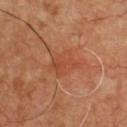Impression: Captured during whole-body skin photography for melanoma surveillance; the lesion was not biopsied. Image and clinical context: Cropped from a total-body skin-imaging series; the visible field is about 15 mm. From the chest. The lesion-visualizer software estimated a lesion area of about 9 mm² and two-axis asymmetry of about 0.4. It also reported an average lesion color of about L≈48 a*≈27 b*≈35 (CIELAB) and a lesion–skin lightness drop of about 6. It also reported a border-irregularity rating of about 4.5/10. The patient is a male aged around 50. Imaged with cross-polarized lighting. Measured at roughly 4.5 mm in maximum diameter.